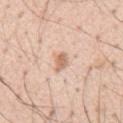Acquisition and patient details: This is a white-light tile. The total-body-photography lesion software estimated a nevus-likeness score of about 85/100. The recorded lesion diameter is about 3 mm. A male subject, approximately 55 years of age. A 15 mm crop from a total-body photograph taken for skin-cancer surveillance. On the back.